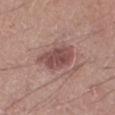{"site": "left lower leg", "image": {"source": "total-body photography crop", "field_of_view_mm": 15}, "automated_metrics": {"cielab_L": 49, "cielab_a": 22, "cielab_b": 21, "vs_skin_darker_L": 11.0, "vs_skin_contrast_norm": 8.0, "color_variation_0_10": 4.0, "peripheral_color_asymmetry": 1.0, "nevus_likeness_0_100": 50, "lesion_detection_confidence_0_100": 100}, "lesion_size": {"long_diameter_mm_approx": 5.5}, "lighting": "white-light", "patient": {"sex": "male", "age_approx": 20}}